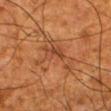The lesion was photographed on a routine skin check and not biopsied; there is no pathology result. A 15 mm close-up tile from a total-body photography series done for melanoma screening. Measured at roughly 7 mm in maximum diameter. A male subject aged approximately 80. From the leg.